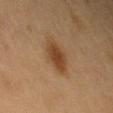This lesion was catalogued during total-body skin photography and was not selected for biopsy.
A region of skin cropped from a whole-body photographic capture, roughly 15 mm wide.
A male subject aged 58 to 62.
The lesion is located on the left upper arm.
Captured under cross-polarized illumination.
Longest diameter approximately 4.5 mm.
The lesion-visualizer software estimated an area of roughly 8 mm², a shape eccentricity near 0.85, and a shape-asymmetry score of about 0.15 (0 = symmetric). The analysis additionally found a border-irregularity index near 2.5/10, a color-variation rating of about 2.5/10, and radial color variation of about 0.5. It also reported a classifier nevus-likeness of about 95/100 and a detector confidence of about 100 out of 100 that the crop contains a lesion.